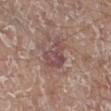workup: total-body-photography surveillance lesion; no biopsy
imaging modality: ~15 mm tile from a whole-body skin photo
patient: male, about 80 years old
lesion size: ~4 mm (longest diameter)
automated lesion analysis: an area of roughly 8.5 mm², a shape eccentricity near 0.7, and two-axis asymmetry of about 0.4; a nevus-likeness score of about 0/100 and a lesion-detection confidence of about 100/100
site: the right lower leg